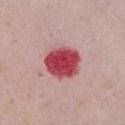Q: Was a biopsy performed?
A: no biopsy performed (imaged during a skin exam)
Q: Lesion location?
A: the chest
Q: Patient demographics?
A: female, aged 38 to 42
Q: What lighting was used for the tile?
A: white-light illumination
Q: What kind of image is this?
A: ~15 mm crop, total-body skin-cancer survey
Q: What did automated image analysis measure?
A: a border-irregularity rating of about 1.5/10, a color-variation rating of about 5/10, and peripheral color asymmetry of about 1.5